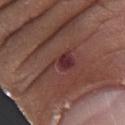• follow-up: no biopsy performed (imaged during a skin exam)
• patient: male, aged 63 to 67
• acquisition: total-body-photography crop, ~15 mm field of view
• tile lighting: white-light illumination
• anatomic site: the arm
• automated metrics: a lesion area of about 6 mm² and a shape eccentricity near 0.85; a border-irregularity index near 6.5/10, internal color variation of about 2 on a 0–10 scale, and peripheral color asymmetry of about 0.5; an automated nevus-likeness rating near 0 out of 100